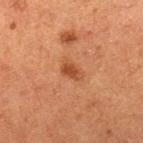Part of a total-body skin-imaging series; this lesion was reviewed on a skin check and was not flagged for biopsy.
On the right lower leg.
About 2.5 mm across.
A lesion tile, about 15 mm wide, cut from a 3D total-body photograph.
A female subject in their 50s.
An algorithmic analysis of the crop reported a border-irregularity rating of about 2.5/10, a within-lesion color-variation index near 1/10, and radial color variation of about 0.5.